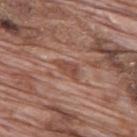<tbp_lesion>
  <image>
    <source>total-body photography crop</source>
    <field_of_view_mm>15</field_of_view_mm>
  </image>
  <lighting>white-light</lighting>
  <lesion_size>
    <long_diameter_mm_approx>3.0</long_diameter_mm_approx>
  </lesion_size>
  <site>mid back</site>
  <patient>
    <sex>male</sex>
    <age_approx>70</age_approx>
  </patient>
</tbp_lesion>